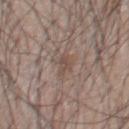Imaged during a routine full-body skin examination; the lesion was not biopsied and no histopathology is available. Imaged with white-light lighting. An algorithmic analysis of the crop reported an area of roughly 3 mm², an outline eccentricity of about 0.75 (0 = round, 1 = elongated), and a shape-asymmetry score of about 0.35 (0 = symmetric). And it measured a nevus-likeness score of about 10/100. A 15 mm crop from a total-body photograph taken for skin-cancer surveillance. Approximately 2.5 mm at its widest. On the chest. The subject is a male in their mid- to late 50s.